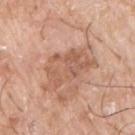Assessment: Part of a total-body skin-imaging series; this lesion was reviewed on a skin check and was not flagged for biopsy. Context: The recorded lesion diameter is about 6.5 mm. Automated tile analysis of the lesion measured a lesion area of about 16 mm², an eccentricity of roughly 0.8, and a shape-asymmetry score of about 0.35 (0 = symmetric). The software also gave a lesion color around L≈58 a*≈22 b*≈31 in CIELAB, roughly 9 lightness units darker than nearby skin, and a lesion-to-skin contrast of about 6 (normalized; higher = more distinct). The software also gave a color-variation rating of about 4.5/10 and a peripheral color-asymmetry measure near 1.5. A male patient aged around 75. A close-up tile cropped from a whole-body skin photograph, about 15 mm across. Imaged with white-light lighting. On the front of the torso.